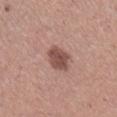notes: no biopsy performed (imaged during a skin exam); anatomic site: the right lower leg; subject: female, in their 30s; acquisition: ~15 mm crop, total-body skin-cancer survey.